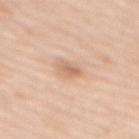The lesion was photographed on a routine skin check and not biopsied; there is no pathology result.
The total-body-photography lesion software estimated an area of roughly 3.5 mm², a shape eccentricity near 0.85, and two-axis asymmetry of about 0.25.
The subject is a female aged approximately 70.
Longest diameter approximately 2.5 mm.
From the mid back.
This is a white-light tile.
A roughly 15 mm field-of-view crop from a total-body skin photograph.